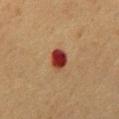Assessment:
The lesion was tiled from a total-body skin photograph and was not biopsied.